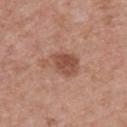The lesion was tiled from a total-body skin photograph and was not biopsied.
A roughly 15 mm field-of-view crop from a total-body skin photograph.
The patient is a male approximately 65 years of age.
Captured under white-light illumination.
The lesion-visualizer software estimated a mean CIELAB color near L≈51 a*≈22 b*≈29, about 10 CIELAB-L* units darker than the surrounding skin, and a normalized lesion–skin contrast near 7.5.
Located on the upper back.